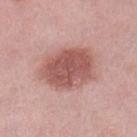biopsy status: imaged on a skin check; not biopsied | patient: female, approximately 40 years of age | image source: 15 mm crop, total-body photography | body site: the left lower leg.